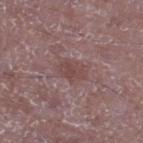Clinical summary: The subject is a male approximately 50 years of age. This is a white-light tile. Automated image analysis of the tile measured a footprint of about 5 mm², an eccentricity of roughly 0.7, and two-axis asymmetry of about 0.25. The analysis additionally found an average lesion color of about L≈45 a*≈19 b*≈19 (CIELAB), roughly 7 lightness units darker than nearby skin, and a normalized border contrast of about 6. It also reported an automated nevus-likeness rating near 0 out of 100 and lesion-presence confidence of about 100/100. The lesion is on the left lower leg. A region of skin cropped from a whole-body photographic capture, roughly 15 mm wide.